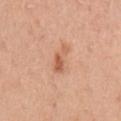Clinical impression:
The lesion was tiled from a total-body skin photograph and was not biopsied.
Clinical summary:
On the left upper arm. Cropped from a total-body skin-imaging series; the visible field is about 15 mm. A female subject, in their mid- to late 40s.